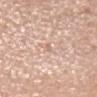The lesion is on the head or neck. A roughly 15 mm field-of-view crop from a total-body skin photograph. Captured under white-light illumination. The patient is a female aged 63 to 67. About 1.5 mm across.